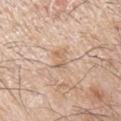Impression: The lesion was photographed on a routine skin check and not biopsied; there is no pathology result. Context: The total-body-photography lesion software estimated a lesion area of about 4 mm², an outline eccentricity of about 0.8 (0 = round, 1 = elongated), and a symmetry-axis asymmetry near 0.25. The software also gave roughly 8 lightness units darker than nearby skin and a normalized border contrast of about 5.5. It also reported a border-irregularity rating of about 2.5/10. The software also gave a nevus-likeness score of about 0/100. A close-up tile cropped from a whole-body skin photograph, about 15 mm across. From the arm. A male subject aged approximately 65. Captured under white-light illumination. The lesion's longest dimension is about 3 mm.